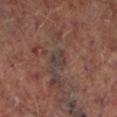No biopsy was performed on this lesion — it was imaged during a full skin examination and was not determined to be concerning.
The tile uses cross-polarized illumination.
On the left lower leg.
A male patient aged approximately 65.
Approximately 2.5 mm at its widest.
Cropped from a whole-body photographic skin survey; the tile spans about 15 mm.
The lesion-visualizer software estimated an automated nevus-likeness rating near 0 out of 100 and lesion-presence confidence of about 60/100.